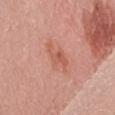A 15 mm close-up extracted from a 3D total-body photography capture. A female patient, aged 38 to 42. Located on the leg. Captured under white-light illumination.